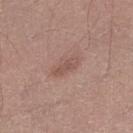biopsy_status: not biopsied; imaged during a skin examination
patient:
  sex: male
  age_approx: 30
lighting: white-light
lesion_size:
  long_diameter_mm_approx: 3.5
image:
  source: total-body photography crop
  field_of_view_mm: 15